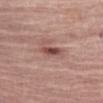Imaged during a routine full-body skin examination; the lesion was not biopsied and no histopathology is available.
This image is a 15 mm lesion crop taken from a total-body photograph.
About 3 mm across.
Automated image analysis of the tile measured a lesion area of about 3.5 mm², an outline eccentricity of about 0.9 (0 = round, 1 = elongated), and a symmetry-axis asymmetry near 0.2. It also reported a lesion color around L≈47 a*≈23 b*≈24 in CIELAB, a lesion–skin lightness drop of about 13, and a normalized lesion–skin contrast near 9.5.
This is a white-light tile.
A female subject, aged 68–72.
The lesion is located on the left thigh.